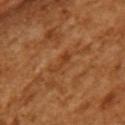workup — catalogued during a skin exam; not biopsied | automated metrics — a lesion color around L≈41 a*≈25 b*≈39 in CIELAB and a lesion–skin lightness drop of about 7; a border-irregularity index near 6/10 and internal color variation of about 0 on a 0–10 scale; a nevus-likeness score of about 0/100 and a detector confidence of about 95 out of 100 that the crop contains a lesion | patient — female, in their mid- to late 50s | acquisition — ~15 mm tile from a whole-body skin photo | illumination — cross-polarized | lesion diameter — about 3 mm | site — the left upper arm.